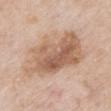biopsy status=no biopsy performed (imaged during a skin exam) | diameter=about 9 mm | patient=male, in their mid- to late 80s or older | anatomic site=the mid back | image=~15 mm tile from a whole-body skin photo | illumination=white-light | automated metrics=an area of roughly 35 mm²; an average lesion color of about L≈61 a*≈18 b*≈30 (CIELAB), a lesion–skin lightness drop of about 12, and a normalized border contrast of about 7.5; border irregularity of about 3.5 on a 0–10 scale, internal color variation of about 7 on a 0–10 scale, and a peripheral color-asymmetry measure near 2.5.